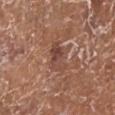The lesion was tiled from a total-body skin photograph and was not biopsied. Automated image analysis of the tile measured border irregularity of about 4 on a 0–10 scale, a within-lesion color-variation index near 6/10, and a peripheral color-asymmetry measure near 2.5. And it measured a detector confidence of about 95 out of 100 that the crop contains a lesion. The patient is a female in their mid-70s. From the left lower leg. A 15 mm close-up tile from a total-body photography series done for melanoma screening. This is a white-light tile. About 3.5 mm across.